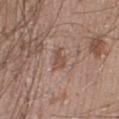The lesion was photographed on a routine skin check and not biopsied; there is no pathology result. The recorded lesion diameter is about 2.5 mm. The total-body-photography lesion software estimated a lesion area of about 4 mm², an eccentricity of roughly 0.7, and two-axis asymmetry of about 0.3. The analysis additionally found an average lesion color of about L≈51 a*≈19 b*≈26 (CIELAB), roughly 7 lightness units darker than nearby skin, and a lesion-to-skin contrast of about 6 (normalized; higher = more distinct). And it measured a classifier nevus-likeness of about 0/100 and a detector confidence of about 100 out of 100 that the crop contains a lesion. The subject is a male roughly 20 years of age. This image is a 15 mm lesion crop taken from a total-body photograph. From the right lower leg. This is a white-light tile.